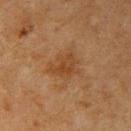Captured during whole-body skin photography for melanoma surveillance; the lesion was not biopsied. Measured at roughly 3.5 mm in maximum diameter. An algorithmic analysis of the crop reported an outline eccentricity of about 0.6 (0 = round, 1 = elongated) and a shape-asymmetry score of about 0.45 (0 = symmetric). Imaged with cross-polarized lighting. Located on the left upper arm. A male subject aged approximately 60. A lesion tile, about 15 mm wide, cut from a 3D total-body photograph.